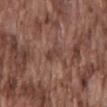Captured during whole-body skin photography for melanoma surveillance; the lesion was not biopsied.
This is a white-light tile.
On the mid back.
Measured at roughly 3 mm in maximum diameter.
A male subject, approximately 75 years of age.
A region of skin cropped from a whole-body photographic capture, roughly 15 mm wide.